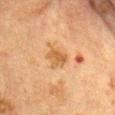Impression: Recorded during total-body skin imaging; not selected for excision or biopsy. Image and clinical context: A 15 mm crop from a total-body photograph taken for skin-cancer surveillance. From the abdomen. The patient is a female aged 48–52.